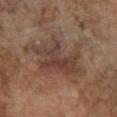The lesion was photographed on a routine skin check and not biopsied; there is no pathology result. A close-up tile cropped from a whole-body skin photograph, about 15 mm across. A male subject approximately 75 years of age. Imaged with white-light lighting. The lesion is on the chest. An algorithmic analysis of the crop reported a lesion area of about 22 mm², an outline eccentricity of about 0.8 (0 = round, 1 = elongated), and a shape-asymmetry score of about 0.4 (0 = symmetric). The analysis additionally found a mean CIELAB color near L≈41 a*≈17 b*≈24 and a lesion–skin lightness drop of about 8. And it measured border irregularity of about 6 on a 0–10 scale and a within-lesion color-variation index near 5.5/10.